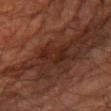Part of a total-body skin-imaging series; this lesion was reviewed on a skin check and was not flagged for biopsy.
A male patient, in their 60s.
The tile uses cross-polarized illumination.
On the leg.
Longest diameter approximately 5.5 mm.
A region of skin cropped from a whole-body photographic capture, roughly 15 mm wide.